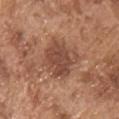The lesion is on the chest.
The lesion's longest dimension is about 5 mm.
This is a white-light tile.
A close-up tile cropped from a whole-body skin photograph, about 15 mm across.
A male patient, aged 73–77.